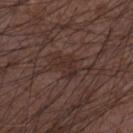No biopsy was performed on this lesion — it was imaged during a full skin examination and was not determined to be concerning. A male patient aged approximately 50. The total-body-photography lesion software estimated a within-lesion color-variation index near 1.5/10 and peripheral color asymmetry of about 0.5. Approximately 3.5 mm at its widest. Imaged with white-light lighting. A 15 mm crop from a total-body photograph taken for skin-cancer surveillance. The lesion is located on the left forearm.